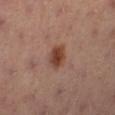Located on the left lower leg.
The tile uses cross-polarized illumination.
Cropped from a whole-body photographic skin survey; the tile spans about 15 mm.
A male subject, approximately 55 years of age.